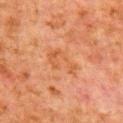Part of a total-body skin-imaging series; this lesion was reviewed on a skin check and was not flagged for biopsy. A region of skin cropped from a whole-body photographic capture, roughly 15 mm wide. Approximately 4.5 mm at its widest. A male patient aged around 80. Captured under cross-polarized illumination. On the right upper arm. Automated tile analysis of the lesion measured an area of roughly 8.5 mm² and an eccentricity of roughly 0.85. The analysis additionally found a lesion color around L≈46 a*≈22 b*≈34 in CIELAB and roughly 5 lightness units darker than nearby skin. And it measured border irregularity of about 4.5 on a 0–10 scale and internal color variation of about 3.5 on a 0–10 scale.